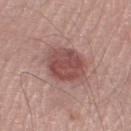Q: Is there a histopathology result?
A: catalogued during a skin exam; not biopsied
Q: Automated lesion metrics?
A: a mean CIELAB color near L≈48 a*≈24 b*≈23; a border-irregularity rating of about 1/10, internal color variation of about 3.5 on a 0–10 scale, and a peripheral color-asymmetry measure near 1
Q: Who is the patient?
A: male, aged 43 to 47
Q: Where on the body is the lesion?
A: the left lower leg
Q: What kind of image is this?
A: total-body-photography crop, ~15 mm field of view